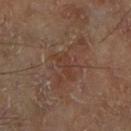Notes:
- notes · no biopsy performed (imaged during a skin exam)
- TBP lesion metrics · an average lesion color of about L≈36 a*≈19 b*≈25 (CIELAB), roughly 5 lightness units darker than nearby skin, and a normalized lesion–skin contrast near 5; a nevus-likeness score of about 0/100 and a detector confidence of about 95 out of 100 that the crop contains a lesion
- image source · ~15 mm tile from a whole-body skin photo
- site · the leg
- lesion size · ~3.5 mm (longest diameter)
- lighting · cross-polarized
- patient · male, roughly 70 years of age